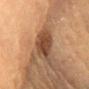Findings:
- lesion size — about 4.5 mm
- anatomic site — the chest
- automated lesion analysis — a lesion area of about 9.5 mm², an outline eccentricity of about 0.75 (0 = round, 1 = elongated), and two-axis asymmetry of about 0.25
- subject — female, in their 60s
- acquisition — total-body-photography crop, ~15 mm field of view
- illumination — cross-polarized illumination